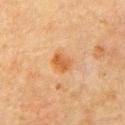Findings:
* lighting — cross-polarized
* image-analysis metrics — an outline eccentricity of about 0.4 (0 = round, 1 = elongated) and a symmetry-axis asymmetry near 0.25; border irregularity of about 2 on a 0–10 scale, a color-variation rating of about 3/10, and peripheral color asymmetry of about 1
* imaging modality — 15 mm crop, total-body photography
* subject — male, roughly 80 years of age
* diameter — ~2.5 mm (longest diameter)
* location — the front of the torso